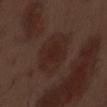follow-up: total-body-photography surveillance lesion; no biopsy
acquisition: 15 mm crop, total-body photography
tile lighting: white-light illumination
TBP lesion metrics: an area of roughly 20 mm² and a shape-asymmetry score of about 0.15 (0 = symmetric); border irregularity of about 2 on a 0–10 scale; an automated nevus-likeness rating near 40 out of 100
lesion size: about 6 mm
anatomic site: the abdomen
subject: male, aged around 70